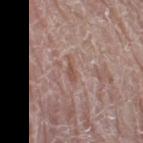No biopsy was performed on this lesion — it was imaged during a full skin examination and was not determined to be concerning. A region of skin cropped from a whole-body photographic capture, roughly 15 mm wide. The lesion is located on the right thigh. A male patient aged approximately 80.